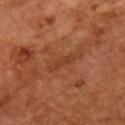Imaged during a routine full-body skin examination; the lesion was not biopsied and no histopathology is available. Cropped from a total-body skin-imaging series; the visible field is about 15 mm. The lesion is on the chest. A male subject aged around 55.